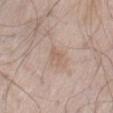Q: Was this lesion biopsied?
A: total-body-photography surveillance lesion; no biopsy
Q: What are the patient's age and sex?
A: male, aged approximately 70
Q: What is the anatomic site?
A: the right thigh
Q: Illumination type?
A: white-light illumination
Q: What did automated image analysis measure?
A: an average lesion color of about L≈60 a*≈15 b*≈27 (CIELAB) and roughly 7 lightness units darker than nearby skin
Q: How large is the lesion?
A: about 2.5 mm
Q: What kind of image is this?
A: ~15 mm crop, total-body skin-cancer survey